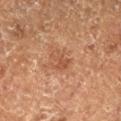– biopsy status · catalogued during a skin exam; not biopsied
– image · total-body-photography crop, ~15 mm field of view
– body site · the left lower leg
– patient · male, roughly 75 years of age
– size · ≈3 mm